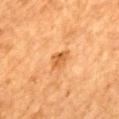The lesion is located on the mid back. A female subject aged approximately 55. Approximately 3 mm at its widest. Cropped from a total-body skin-imaging series; the visible field is about 15 mm. Imaged with cross-polarized lighting.